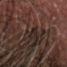follow-up = total-body-photography surveillance lesion; no biopsy | automated metrics = two-axis asymmetry of about 0.35; a mean CIELAB color near L≈20 a*≈11 b*≈16, about 5 CIELAB-L* units darker than the surrounding skin, and a normalized border contrast of about 6.5 | subject = male, about 55 years old | tile lighting = cross-polarized | size = about 3.5 mm | image source = total-body-photography crop, ~15 mm field of view | location = the head or neck.